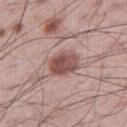| feature | finding |
|---|---|
| workup | imaged on a skin check; not biopsied |
| image | total-body-photography crop, ~15 mm field of view |
| diameter | about 4 mm |
| body site | the right thigh |
| subject | male, aged 58 to 62 |